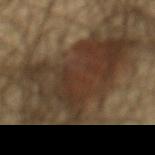Q: Is there a histopathology result?
A: total-body-photography surveillance lesion; no biopsy
Q: Illumination type?
A: cross-polarized illumination
Q: Lesion size?
A: about 12 mm
Q: What is the imaging modality?
A: ~15 mm tile from a whole-body skin photo
Q: Who is the patient?
A: male, aged 43–47
Q: Lesion location?
A: the lower back
Q: What did automated image analysis measure?
A: a border-irregularity index near 8/10, a color-variation rating of about 6/10, and peripheral color asymmetry of about 2